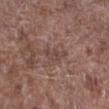The patient is a male aged 53–57. Captured under white-light illumination. A lesion tile, about 15 mm wide, cut from a 3D total-body photograph. Automated tile analysis of the lesion measured a nevus-likeness score of about 0/100. The lesion is located on the right lower leg. Measured at roughly 3.5 mm in maximum diameter.